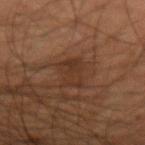Captured during whole-body skin photography for melanoma surveillance; the lesion was not biopsied.
The total-body-photography lesion software estimated an average lesion color of about L≈30 a*≈17 b*≈26 (CIELAB), a lesion–skin lightness drop of about 5, and a lesion-to-skin contrast of about 5.5 (normalized; higher = more distinct). The software also gave a detector confidence of about 95 out of 100 that the crop contains a lesion.
A male patient, in their mid- to late 50s.
Located on the left forearm.
A region of skin cropped from a whole-body photographic capture, roughly 15 mm wide.